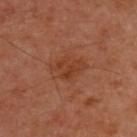  biopsy_status: not biopsied; imaged during a skin examination
  patient:
    sex: male
    age_approx: 50
  lesion_size:
    long_diameter_mm_approx: 3.5
  site: back
  image:
    source: total-body photography crop
    field_of_view_mm: 15
  lighting: cross-polarized
  automated_metrics:
    eccentricity: 0.7
    shape_asymmetry: 0.35
    vs_skin_darker_L: 7.0
    vs_skin_contrast_norm: 6.0
    border_irregularity_0_10: 4.5
    color_variation_0_10: 3.0
    peripheral_color_asymmetry: 1.0